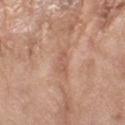| feature | finding |
|---|---|
| workup | total-body-photography surveillance lesion; no biopsy |
| location | the left forearm |
| patient | female, in their mid-70s |
| image | 15 mm crop, total-body photography |